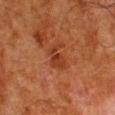Recorded during total-body skin imaging; not selected for excision or biopsy. A male patient roughly 80 years of age. Cropped from a total-body skin-imaging series; the visible field is about 15 mm. Automated tile analysis of the lesion measured a lesion area of about 6 mm², an outline eccentricity of about 0.75 (0 = round, 1 = elongated), and a symmetry-axis asymmetry near 0.4. And it measured an average lesion color of about L≈30 a*≈24 b*≈30 (CIELAB). And it measured a border-irregularity index near 5/10, a within-lesion color-variation index near 2/10, and peripheral color asymmetry of about 0.5. Longest diameter approximately 3.5 mm. The lesion is located on the right lower leg.